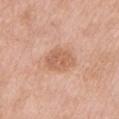Q: Is there a histopathology result?
A: total-body-photography surveillance lesion; no biopsy
Q: What kind of image is this?
A: total-body-photography crop, ~15 mm field of view
Q: Patient demographics?
A: female, in their mid-50s
Q: Automated lesion metrics?
A: an average lesion color of about L≈61 a*≈22 b*≈33 (CIELAB), roughly 9 lightness units darker than nearby skin, and a normalized lesion–skin contrast near 6.5; internal color variation of about 2.5 on a 0–10 scale and radial color variation of about 1; an automated nevus-likeness rating near 10 out of 100 and a detector confidence of about 100 out of 100 that the crop contains a lesion
Q: Illumination type?
A: white-light
Q: Lesion size?
A: ~3.5 mm (longest diameter)
Q: Where on the body is the lesion?
A: the left upper arm